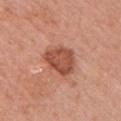Q: How was this image acquired?
A: ~15 mm crop, total-body skin-cancer survey
Q: Patient demographics?
A: female, aged 53 to 57
Q: Lesion size?
A: ~4.5 mm (longest diameter)
Q: Illumination type?
A: white-light
Q: Lesion location?
A: the left upper arm